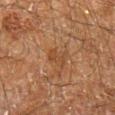Imaged during a routine full-body skin examination; the lesion was not biopsied and no histopathology is available. The lesion-visualizer software estimated a lesion color around L≈35 a*≈17 b*≈28 in CIELAB, about 5 CIELAB-L* units darker than the surrounding skin, and a normalized border contrast of about 5. It also reported border irregularity of about 4 on a 0–10 scale, internal color variation of about 2 on a 0–10 scale, and a peripheral color-asymmetry measure near 0.5. A 15 mm close-up tile from a total-body photography series done for melanoma screening. The recorded lesion diameter is about 3 mm. A male patient, approximately 60 years of age. Captured under cross-polarized illumination. The lesion is on the leg.